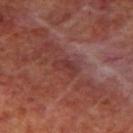Notes:
– workup: no biopsy performed (imaged during a skin exam)
– lesion diameter: about 3 mm
– acquisition: ~15 mm crop, total-body skin-cancer survey
– subject: male, about 70 years old
– site: the mid back
– tile lighting: cross-polarized